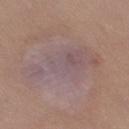| key | value |
|---|---|
| notes | total-body-photography surveillance lesion; no biopsy |
| lesion diameter | ~10.5 mm (longest diameter) |
| lighting | white-light |
| subject | female, in their 20s |
| image source | total-body-photography crop, ~15 mm field of view |
| location | the right thigh |